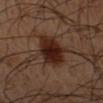Q: Is there a histopathology result?
A: imaged on a skin check; not biopsied
Q: What is the lesion's diameter?
A: ≈5 mm
Q: Automated lesion metrics?
A: a border-irregularity index near 2/10
Q: What is the anatomic site?
A: the left forearm
Q: How was the tile lit?
A: cross-polarized illumination
Q: What is the imaging modality?
A: ~15 mm tile from a whole-body skin photo
Q: Patient demographics?
A: male, roughly 50 years of age